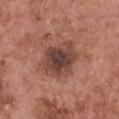{
  "biopsy_status": "not biopsied; imaged during a skin examination",
  "site": "head or neck",
  "automated_metrics": {
    "eccentricity": 0.8,
    "border_irregularity_0_10": 2.5,
    "color_variation_0_10": 6.0,
    "peripheral_color_asymmetry": 1.5
  },
  "image": {
    "source": "total-body photography crop",
    "field_of_view_mm": 15
  },
  "patient": {
    "sex": "male",
    "age_approx": 50
  },
  "lesion_size": {
    "long_diameter_mm_approx": 6.0
  }
}